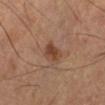notes: total-body-photography surveillance lesion; no biopsy
illumination: cross-polarized
patient: male, about 60 years old
imaging modality: ~15 mm tile from a whole-body skin photo
body site: the leg
lesion size: ≈2.5 mm